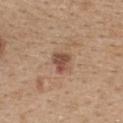Background:
The lesion is located on the upper back. Approximately 3 mm at its widest. A roughly 15 mm field-of-view crop from a total-body skin photograph. A female patient, in their mid-40s.